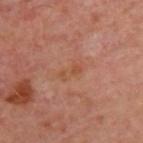Part of a total-body skin-imaging series; this lesion was reviewed on a skin check and was not flagged for biopsy. From the back. Automated tile analysis of the lesion measured a lesion area of about 4 mm², an outline eccentricity of about 0.9 (0 = round, 1 = elongated), and a symmetry-axis asymmetry near 0.2. It also reported about 5 CIELAB-L* units darker than the surrounding skin and a normalized border contrast of about 5.5. And it measured a nevus-likeness score of about 0/100 and a detector confidence of about 100 out of 100 that the crop contains a lesion. Measured at roughly 3.5 mm in maximum diameter. A roughly 15 mm field-of-view crop from a total-body skin photograph. Imaged with cross-polarized lighting. A subject in their mid-50s.